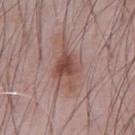Q: Was this lesion biopsied?
A: no biopsy performed (imaged during a skin exam)
Q: What is the imaging modality?
A: total-body-photography crop, ~15 mm field of view
Q: How large is the lesion?
A: about 5.5 mm
Q: Lesion location?
A: the abdomen
Q: Who is the patient?
A: male, aged 63 to 67